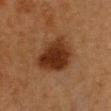The lesion was photographed on a routine skin check and not biopsied; there is no pathology result. Captured under cross-polarized illumination. The patient is a female aged 38–42. On the chest. Approximately 5.5 mm at its widest. A 15 mm close-up tile from a total-body photography series done for melanoma screening.